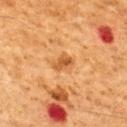No biopsy was performed on this lesion — it was imaged during a full skin examination and was not determined to be concerning. Cropped from a whole-body photographic skin survey; the tile spans about 15 mm. This is a cross-polarized tile. A male subject aged around 60. Approximately 2.5 mm at its widest. The lesion is on the upper back.